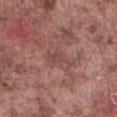biopsy status = total-body-photography surveillance lesion; no biopsy | anatomic site = the abdomen | subject = male, aged 73–77 | lighting = white-light | size = ≈3.5 mm | image = ~15 mm crop, total-body skin-cancer survey.